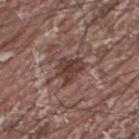The lesion was tiled from a total-body skin photograph and was not biopsied. A male patient approximately 40 years of age. A 15 mm close-up tile from a total-body photography series done for melanoma screening. Approximately 4 mm at its widest. On the upper back. The tile uses white-light illumination. Automated tile analysis of the lesion measured a border-irregularity index near 3.5/10 and a color-variation rating of about 3.5/10. It also reported a classifier nevus-likeness of about 15/100 and a detector confidence of about 60 out of 100 that the crop contains a lesion.